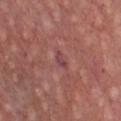biopsy status=total-body-photography surveillance lesion; no biopsy | tile lighting=white-light illumination | anatomic site=the chest | image source=total-body-photography crop, ~15 mm field of view | lesion size=about 2.5 mm | patient=male, aged approximately 60.